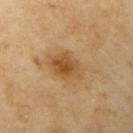Notes:
* workup: imaged on a skin check; not biopsied
* anatomic site: the left upper arm
* acquisition: total-body-photography crop, ~15 mm field of view
* patient: female, roughly 70 years of age
* lesion diameter: ≈4.5 mm